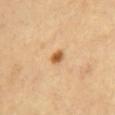The lesion was photographed on a routine skin check and not biopsied; there is no pathology result. On the chest. A 15 mm close-up extracted from a 3D total-body photography capture. The patient is a female aged around 70.